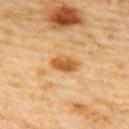Impression:
Recorded during total-body skin imaging; not selected for excision or biopsy.
Acquisition and patient details:
A female patient, approximately 60 years of age. The total-body-photography lesion software estimated a footprint of about 5.5 mm², an eccentricity of roughly 0.85, and a shape-asymmetry score of about 0.2 (0 = symmetric). It also reported an automated nevus-likeness rating near 95 out of 100 and a detector confidence of about 100 out of 100 that the crop contains a lesion. A 15 mm crop from a total-body photograph taken for skin-cancer surveillance. The lesion is on the upper back. The recorded lesion diameter is about 3.5 mm.